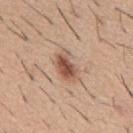Captured during whole-body skin photography for melanoma surveillance; the lesion was not biopsied. Imaged with white-light lighting. A 15 mm crop from a total-body photograph taken for skin-cancer surveillance. The lesion is located on the mid back. About 3.5 mm across. A male subject, aged approximately 60.